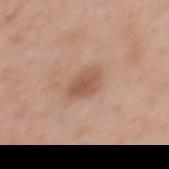follow-up: no biopsy performed (imaged during a skin exam)
image source: total-body-photography crop, ~15 mm field of view
diameter: ≈3.5 mm
image-analysis metrics: a footprint of about 7 mm², an eccentricity of roughly 0.75, and a shape-asymmetry score of about 0.2 (0 = symmetric); border irregularity of about 2 on a 0–10 scale, a color-variation rating of about 2.5/10, and radial color variation of about 1
body site: the upper back
subject: female, aged approximately 50